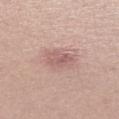Part of a total-body skin-imaging series; this lesion was reviewed on a skin check and was not flagged for biopsy. A 15 mm close-up tile from a total-body photography series done for melanoma screening. A female patient, aged approximately 20. Automated image analysis of the tile measured an area of roughly 6.5 mm², a shape eccentricity near 0.7, and two-axis asymmetry of about 0.25. It also reported a border-irregularity index near 2.5/10, a color-variation rating of about 3.5/10, and radial color variation of about 1. It also reported a classifier nevus-likeness of about 0/100 and lesion-presence confidence of about 100/100. The lesion is on the left lower leg. Captured under white-light illumination. The lesion's longest dimension is about 3.5 mm.